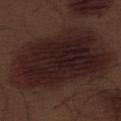Imaged with white-light lighting. On the abdomen. Automated tile analysis of the lesion measured a shape eccentricity near 0.85. It also reported a border-irregularity rating of about 2.5/10, a color-variation rating of about 4.5/10, and peripheral color asymmetry of about 1.5. A male subject, aged 68–72. A 15 mm close-up extracted from a 3D total-body photography capture.